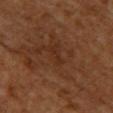Case summary:
- follow-up: no biopsy performed (imaged during a skin exam)
- tile lighting: cross-polarized illumination
- location: the chest
- patient: male, aged 58–62
- TBP lesion metrics: an area of roughly 70 mm² and a symmetry-axis asymmetry near 0.55; a border-irregularity index near 9/10, a color-variation rating of about 4/10, and peripheral color asymmetry of about 1
- lesion diameter: ≈17 mm
- image: total-body-photography crop, ~15 mm field of view